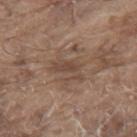| field | value |
|---|---|
| workup | total-body-photography surveillance lesion; no biopsy |
| subject | male, roughly 80 years of age |
| lighting | white-light |
| lesion size | about 4 mm |
| image source | ~15 mm tile from a whole-body skin photo |
| site | the mid back |